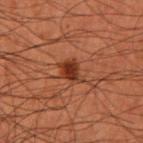biopsy status: total-body-photography surveillance lesion; no biopsy.